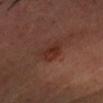follow-up: imaged on a skin check; not biopsied | imaging modality: ~15 mm tile from a whole-body skin photo | anatomic site: the head or neck | lesion diameter: about 3.5 mm | patient: male, aged 43 to 47 | tile lighting: cross-polarized illumination.